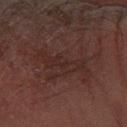Recorded during total-body skin imaging; not selected for excision or biopsy. Automated image analysis of the tile measured an eccentricity of roughly 0.95 and two-axis asymmetry of about 0.5. The software also gave a mean CIELAB color near L≈20 a*≈15 b*≈16 and roughly 4 lightness units darker than nearby skin. This is a cross-polarized tile. Approximately 6.5 mm at its widest. Located on the left forearm. The subject is a male approximately 70 years of age. A 15 mm crop from a total-body photograph taken for skin-cancer surveillance.